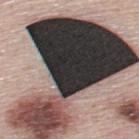Acquisition and patient details: A male subject, aged 43 to 47. A roughly 15 mm field-of-view crop from a total-body skin photograph. This is a white-light tile. Located on the upper back. About 15.5 mm across. Conclusion: On excision, pathology confirmed a dysplastic (Clark) nevus.